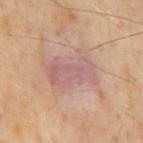  biopsy_status: not biopsied; imaged during a skin examination
  site: back
  patient:
    sex: male
    age_approx: 55
  automated_metrics:
    eccentricity: 0.8
    shape_asymmetry: 0.35
    vs_skin_darker_L: 7.0
    vs_skin_contrast_norm: 6.5
    color_variation_0_10: 3.5
    peripheral_color_asymmetry: 1.0
  image:
    source: total-body photography crop
    field_of_view_mm: 15